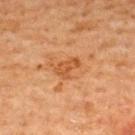Clinical impression:
The lesion was tiled from a total-body skin photograph and was not biopsied.
Acquisition and patient details:
Measured at roughly 3 mm in maximum diameter. The tile uses cross-polarized illumination. A male patient roughly 65 years of age. A close-up tile cropped from a whole-body skin photograph, about 15 mm across. From the upper back.